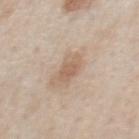Findings:
• lighting · white-light
• location · the chest
• patient · male, roughly 60 years of age
• image · ~15 mm tile from a whole-body skin photo
• image-analysis metrics · a footprint of about 5 mm², an outline eccentricity of about 0.7 (0 = round, 1 = elongated), and a symmetry-axis asymmetry near 0.25; a lesion color around L≈60 a*≈16 b*≈29 in CIELAB, roughly 8 lightness units darker than nearby skin, and a lesion-to-skin contrast of about 6 (normalized; higher = more distinct); a classifier nevus-likeness of about 10/100 and a detector confidence of about 100 out of 100 that the crop contains a lesion